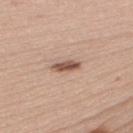Part of a total-body skin-imaging series; this lesion was reviewed on a skin check and was not flagged for biopsy.
The lesion-visualizer software estimated an area of roughly 4 mm², a shape eccentricity near 0.9, and a symmetry-axis asymmetry near 0.3. And it measured an average lesion color of about L≈54 a*≈20 b*≈27 (CIELAB), about 14 CIELAB-L* units darker than the surrounding skin, and a lesion-to-skin contrast of about 9.5 (normalized; higher = more distinct). The analysis additionally found border irregularity of about 3 on a 0–10 scale, a within-lesion color-variation index near 4/10, and peripheral color asymmetry of about 1.5.
Cropped from a whole-body photographic skin survey; the tile spans about 15 mm.
The patient is a female aged 23–27.
The lesion is on the upper back.